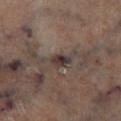{"biopsy_status": "not biopsied; imaged during a skin examination", "image": {"source": "total-body photography crop", "field_of_view_mm": 15}, "lighting": "cross-polarized", "lesion_size": {"long_diameter_mm_approx": 2.5}, "site": "right lower leg", "patient": {"sex": "male", "age_approx": 65}, "automated_metrics": {"eccentricity": 0.8, "nevus_likeness_0_100": 0, "lesion_detection_confidence_0_100": 55}}